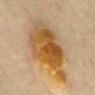The lesion was photographed on a routine skin check and not biopsied; there is no pathology result. An algorithmic analysis of the crop reported a mean CIELAB color near L≈45 a*≈16 b*≈36, about 11 CIELAB-L* units darker than the surrounding skin, and a lesion-to-skin contrast of about 10.5 (normalized; higher = more distinct). And it measured a border-irregularity index near 3.5/10, a color-variation rating of about 8/10, and peripheral color asymmetry of about 2. Cropped from a total-body skin-imaging series; the visible field is about 15 mm. A male subject about 75 years old. The lesion is located on the chest. About 9 mm across.